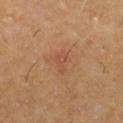notes = catalogued during a skin exam; not biopsied
imaging modality = ~15 mm tile from a whole-body skin photo
patient = male, about 60 years old
anatomic site = the right thigh